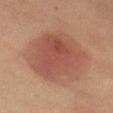The lesion was photographed on a routine skin check and not biopsied; there is no pathology result.
A close-up tile cropped from a whole-body skin photograph, about 15 mm across.
The lesion is located on the left thigh.
The subject is a female aged 58 to 62.